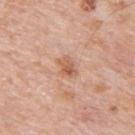Context:
A roughly 15 mm field-of-view crop from a total-body skin photograph. The lesion's longest dimension is about 2.5 mm. Located on the upper back. The subject is a male roughly 80 years of age. Imaged with white-light lighting. The total-body-photography lesion software estimated a footprint of about 4 mm², an outline eccentricity of about 0.75 (0 = round, 1 = elongated), and a symmetry-axis asymmetry near 0.3. It also reported an average lesion color of about L≈59 a*≈23 b*≈33 (CIELAB) and roughly 10 lightness units darker than nearby skin. The software also gave border irregularity of about 3 on a 0–10 scale, a within-lesion color-variation index near 4/10, and radial color variation of about 1.5.